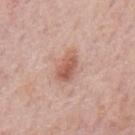{"biopsy_status": "not biopsied; imaged during a skin examination", "lesion_size": {"long_diameter_mm_approx": 3.5}, "image": {"source": "total-body photography crop", "field_of_view_mm": 15}, "lighting": "white-light", "patient": {"sex": "male", "age_approx": 75}, "site": "mid back"}